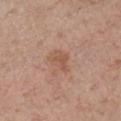This lesion was catalogued during total-body skin photography and was not selected for biopsy.
The lesion is on the chest.
A 15 mm crop from a total-body photograph taken for skin-cancer surveillance.
The tile uses white-light illumination.
A male patient, aged 73–77.
The lesion's longest dimension is about 3 mm.
The lesion-visualizer software estimated a footprint of about 5 mm², an outline eccentricity of about 0.7 (0 = round, 1 = elongated), and a shape-asymmetry score of about 0.4 (0 = symmetric). And it measured about 7 CIELAB-L* units darker than the surrounding skin. It also reported a classifier nevus-likeness of about 0/100.